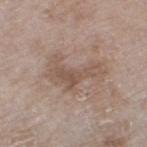  biopsy_status: not biopsied; imaged during a skin examination
  lighting: white-light
  lesion_size:
    long_diameter_mm_approx: 6.5
  patient:
    sex: female
    age_approx: 75
  image:
    source: total-body photography crop
    field_of_view_mm: 15
  site: left thigh